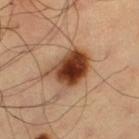biopsy status = total-body-photography surveillance lesion; no biopsy
patient = male, aged approximately 60
site = the left thigh
lesion diameter = ≈5.5 mm
illumination = cross-polarized
automated lesion analysis = a mean CIELAB color near L≈42 a*≈22 b*≈32 and roughly 18 lightness units darker than nearby skin; a border-irregularity rating of about 3/10 and peripheral color asymmetry of about 3.5; a nevus-likeness score of about 100/100 and a lesion-detection confidence of about 100/100
imaging modality = ~15 mm crop, total-body skin-cancer survey